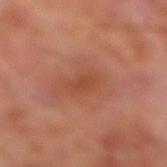Q: Was this lesion biopsied?
A: total-body-photography surveillance lesion; no biopsy
Q: What lighting was used for the tile?
A: cross-polarized
Q: Patient demographics?
A: male, aged approximately 70
Q: What kind of image is this?
A: total-body-photography crop, ~15 mm field of view
Q: What is the anatomic site?
A: the right lower leg